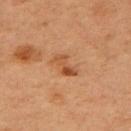notes: catalogued during a skin exam; not biopsied
subject: female, aged around 40
body site: the right upper arm
imaging modality: 15 mm crop, total-body photography